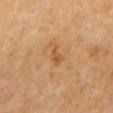biopsy status = catalogued during a skin exam; not biopsied | patient = male, aged around 55 | automated metrics = two-axis asymmetry of about 0.45; a mean CIELAB color near L≈53 a*≈22 b*≈39 and a lesion–skin lightness drop of about 7; a border-irregularity rating of about 4.5/10, a color-variation rating of about 0.5/10, and a peripheral color-asymmetry measure near 0 | image source = total-body-photography crop, ~15 mm field of view | lesion size = about 2.5 mm | body site = the mid back | tile lighting = cross-polarized.